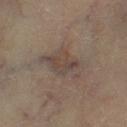<lesion>
  <biopsy_status>not biopsied; imaged during a skin examination</biopsy_status>
  <patient>
    <sex>male</sex>
    <age_approx>70</age_approx>
  </patient>
  <site>left lower leg</site>
  <image>
    <source>total-body photography crop</source>
    <field_of_view_mm>15</field_of_view_mm>
  </image>
  <lesion_size>
    <long_diameter_mm_approx>5.5</long_diameter_mm_approx>
  </lesion_size>
  <lighting>cross-polarized</lighting>
</lesion>